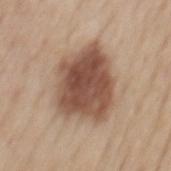A 15 mm close-up tile from a total-body photography series done for melanoma screening.
The subject is a male approximately 65 years of age.
Captured under white-light illumination.
Longest diameter approximately 7.5 mm.
From the mid back.